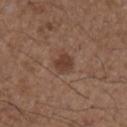Clinical impression:
The lesion was tiled from a total-body skin photograph and was not biopsied.
Acquisition and patient details:
This image is a 15 mm lesion crop taken from a total-body photograph. The lesion is located on the right upper arm. A male subject aged 48 to 52.